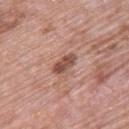Impression:
The lesion was photographed on a routine skin check and not biopsied; there is no pathology result.
Clinical summary:
The lesion's longest dimension is about 3.5 mm. An algorithmic analysis of the crop reported a footprint of about 4.5 mm², an outline eccentricity of about 0.9 (0 = round, 1 = elongated), and a shape-asymmetry score of about 0.2 (0 = symmetric). And it measured a border-irregularity rating of about 2/10 and internal color variation of about 2.5 on a 0–10 scale. Cropped from a total-body skin-imaging series; the visible field is about 15 mm. A female subject, about 60 years old. Imaged with white-light lighting. On the upper back.